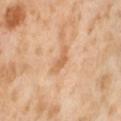notes = no biopsy performed (imaged during a skin exam)
anatomic site = the abdomen
subject = female, about 55 years old
acquisition = ~15 mm crop, total-body skin-cancer survey